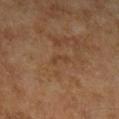Captured during whole-body skin photography for melanoma surveillance; the lesion was not biopsied.
Imaged with cross-polarized lighting.
Automated tile analysis of the lesion measured a footprint of about 2 mm² and a shape-asymmetry score of about 0.45 (0 = symmetric). The software also gave a lesion color around L≈37 a*≈17 b*≈30 in CIELAB, roughly 5 lightness units darker than nearby skin, and a normalized border contrast of about 5. It also reported a border-irregularity index near 4.5/10, internal color variation of about 0 on a 0–10 scale, and radial color variation of about 0. And it measured a nevus-likeness score of about 0/100 and a detector confidence of about 100 out of 100 that the crop contains a lesion.
From the right lower leg.
Approximately 2.5 mm at its widest.
A region of skin cropped from a whole-body photographic capture, roughly 15 mm wide.
The subject is a male in their 60s.